A roughly 15 mm field-of-view crop from a total-body skin photograph; a female subject aged around 45; on the upper back: 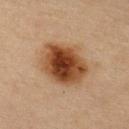Captured under cross-polarized illumination.
The recorded lesion diameter is about 6 mm.
On biopsy, histopathology showed a melanoma in situ, superficial spreading type.